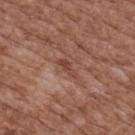The lesion was photographed on a routine skin check and not biopsied; there is no pathology result. A male patient, aged approximately 75. This image is a 15 mm lesion crop taken from a total-body photograph. Imaged with white-light lighting. The lesion is on the upper back.